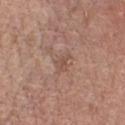Part of a total-body skin-imaging series; this lesion was reviewed on a skin check and was not flagged for biopsy.
A region of skin cropped from a whole-body photographic capture, roughly 15 mm wide.
The patient is a male aged around 60.
From the chest.